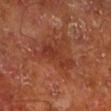Part of a total-body skin-imaging series; this lesion was reviewed on a skin check and was not flagged for biopsy. A male patient in their mid-60s. The lesion is located on the left lower leg. Approximately 4.5 mm at its widest. Captured under cross-polarized illumination. A 15 mm close-up tile from a total-body photography series done for melanoma screening.